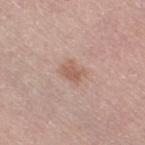Recorded during total-body skin imaging; not selected for excision or biopsy. This is a white-light tile. The patient is a female roughly 65 years of age. A 15 mm crop from a total-body photograph taken for skin-cancer surveillance. On the right thigh.